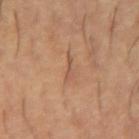Part of a total-body skin-imaging series; this lesion was reviewed on a skin check and was not flagged for biopsy. Longest diameter approximately 2.5 mm. Captured under cross-polarized illumination. The subject is a male aged 53–57. From the arm. A 15 mm close-up tile from a total-body photography series done for melanoma screening. Automated tile analysis of the lesion measured an automated nevus-likeness rating near 0 out of 100 and a detector confidence of about 90 out of 100 that the crop contains a lesion.